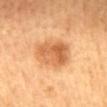biopsy status: imaged on a skin check; not biopsied | subject: female, in their 60s | tile lighting: cross-polarized illumination | lesion size: ≈5 mm | site: the mid back | imaging modality: ~15 mm crop, total-body skin-cancer survey | automated metrics: a lesion color around L≈65 a*≈27 b*≈44 in CIELAB, about 13 CIELAB-L* units darker than the surrounding skin, and a normalized border contrast of about 7.5; a classifier nevus-likeness of about 90/100.